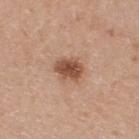Clinical impression:
This lesion was catalogued during total-body skin photography and was not selected for biopsy.
Background:
Longest diameter approximately 3.5 mm. A lesion tile, about 15 mm wide, cut from a 3D total-body photograph. The patient is a female aged around 40. Located on the back. This is a white-light tile.